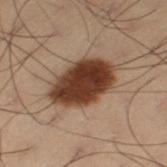  biopsy_status: not biopsied; imaged during a skin examination
  lesion_size:
    long_diameter_mm_approx: 6.5
  lighting: cross-polarized
  image:
    source: total-body photography crop
    field_of_view_mm: 15
  patient:
    sex: male
    age_approx: 55
  site: left thigh